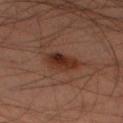follow-up = catalogued during a skin exam; not biopsied | subject = male, aged approximately 45 | lesion size = ≈4 mm | site = the left lower leg | image source = 15 mm crop, total-body photography | automated lesion analysis = a lesion color around L≈29 a*≈21 b*≈26 in CIELAB, roughly 9 lightness units darker than nearby skin, and a normalized border contrast of about 9.5; a border-irregularity index near 2/10 and internal color variation of about 4.5 on a 0–10 scale.